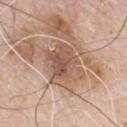Q: Was this lesion biopsied?
A: total-body-photography surveillance lesion; no biopsy
Q: What are the patient's age and sex?
A: male, aged 78 to 82
Q: Lesion size?
A: about 5 mm
Q: What is the anatomic site?
A: the front of the torso
Q: What kind of image is this?
A: ~15 mm crop, total-body skin-cancer survey